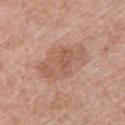The lesion was photographed on a routine skin check and not biopsied; there is no pathology result. From the left upper arm. Measured at roughly 6 mm in maximum diameter. Automated image analysis of the tile measured a footprint of about 18 mm², an outline eccentricity of about 0.8 (0 = round, 1 = elongated), and a symmetry-axis asymmetry near 0.2. The software also gave about 9 CIELAB-L* units darker than the surrounding skin and a lesion-to-skin contrast of about 6 (normalized; higher = more distinct). And it measured a border-irregularity index near 3/10 and internal color variation of about 3.5 on a 0–10 scale. The patient is a female roughly 60 years of age. Cropped from a whole-body photographic skin survey; the tile spans about 15 mm.